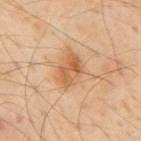workup: imaged on a skin check; not biopsied | image source: total-body-photography crop, ~15 mm field of view | patient: male, approximately 55 years of age | site: the mid back | lesion diameter: about 4 mm | illumination: cross-polarized.